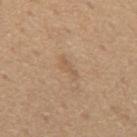<tbp_lesion>
<biopsy_status>not biopsied; imaged during a skin examination</biopsy_status>
<site>mid back</site>
<image>
  <source>total-body photography crop</source>
  <field_of_view_mm>15</field_of_view_mm>
</image>
<lighting>white-light</lighting>
<patient>
  <sex>male</sex>
  <age_approx>65</age_approx>
</patient>
<lesion_size>
  <long_diameter_mm_approx>3.0</long_diameter_mm_approx>
</lesion_size>
</tbp_lesion>